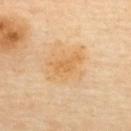follow-up: total-body-photography surveillance lesion; no biopsy
location: the upper back
imaging modality: ~15 mm tile from a whole-body skin photo
image-analysis metrics: border irregularity of about 4 on a 0–10 scale, a color-variation rating of about 2/10, and peripheral color asymmetry of about 0.5; a nevus-likeness score of about 10/100 and a detector confidence of about 100 out of 100 that the crop contains a lesion
subject: female, about 60 years old